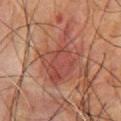Imaged during a routine full-body skin examination; the lesion was not biopsied and no histopathology is available. A lesion tile, about 15 mm wide, cut from a 3D total-body photograph. A male patient, approximately 65 years of age. Approximately 6.5 mm at its widest. From the chest.